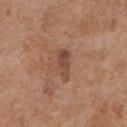workup: total-body-photography surveillance lesion; no biopsy
lesion diameter: ≈4 mm
body site: the chest
patient: female, in their mid-70s
tile lighting: white-light illumination
imaging modality: ~15 mm crop, total-body skin-cancer survey